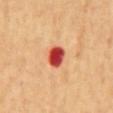This lesion was catalogued during total-body skin photography and was not selected for biopsy. The tile uses cross-polarized illumination. The lesion is on the mid back. The total-body-photography lesion software estimated a border-irregularity rating of about 1.5/10, a within-lesion color-variation index near 5.5/10, and radial color variation of about 2. The analysis additionally found a nevus-likeness score of about 0/100 and lesion-presence confidence of about 100/100. Approximately 2.5 mm at its widest. Cropped from a total-body skin-imaging series; the visible field is about 15 mm. A male subject in their mid- to late 60s.